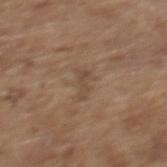This lesion was catalogued during total-body skin photography and was not selected for biopsy.
The lesion's longest dimension is about 3 mm.
An algorithmic analysis of the crop reported a lesion area of about 3.5 mm², a shape eccentricity near 0.9, and a shape-asymmetry score of about 0.5 (0 = symmetric). It also reported a lesion color around L≈47 a*≈16 b*≈28 in CIELAB and roughly 6 lightness units darker than nearby skin. The software also gave a peripheral color-asymmetry measure near 0.
A 15 mm close-up tile from a total-body photography series done for melanoma screening.
Captured under white-light illumination.
A female subject, about 65 years old.
From the mid back.